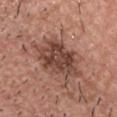Clinical impression:
The lesion was tiled from a total-body skin photograph and was not biopsied.
Background:
Imaged with white-light lighting. A roughly 15 mm field-of-view crop from a total-body skin photograph. Longest diameter approximately 7 mm. The lesion-visualizer software estimated an area of roughly 19 mm². The software also gave roughly 12 lightness units darker than nearby skin and a normalized lesion–skin contrast near 9.5. The analysis additionally found a border-irregularity rating of about 3.5/10, a within-lesion color-variation index near 5/10, and peripheral color asymmetry of about 1.5. It also reported a classifier nevus-likeness of about 30/100. On the head or neck. A male subject, approximately 55 years of age.